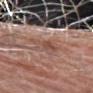| feature | finding |
|---|---|
| biopsy status | catalogued during a skin exam; not biopsied |
| site | the left upper arm |
| imaging modality | ~15 mm crop, total-body skin-cancer survey |
| subject | male, about 80 years old |
| diameter | about 3.5 mm |